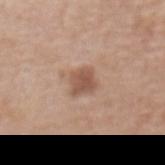Q: Was this lesion biopsied?
A: catalogued during a skin exam; not biopsied
Q: Who is the patient?
A: female, roughly 75 years of age
Q: What kind of image is this?
A: 15 mm crop, total-body photography
Q: What is the anatomic site?
A: the front of the torso
Q: Lesion size?
A: ~2.5 mm (longest diameter)